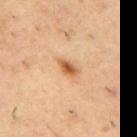Q: Is there a histopathology result?
A: no biopsy performed (imaged during a skin exam)
Q: What lighting was used for the tile?
A: cross-polarized illumination
Q: Who is the patient?
A: male, in their mid-50s
Q: What is the anatomic site?
A: the back
Q: Lesion size?
A: ≈2.5 mm
Q: What is the imaging modality?
A: 15 mm crop, total-body photography
Q: What did automated image analysis measure?
A: an area of roughly 3.5 mm², an outline eccentricity of about 0.85 (0 = round, 1 = elongated), and a shape-asymmetry score of about 0.25 (0 = symmetric)